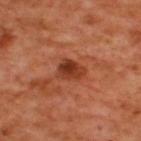Assessment:
The lesion was tiled from a total-body skin photograph and was not biopsied.
Clinical summary:
Longest diameter approximately 3 mm. A 15 mm close-up tile from a total-body photography series done for melanoma screening. Located on the upper back. The patient is a male approximately 50 years of age. Automated image analysis of the tile measured a lesion area of about 6 mm², an eccentricity of roughly 0.7, and a shape-asymmetry score of about 0.25 (0 = symmetric). The analysis additionally found an average lesion color of about L≈38 a*≈29 b*≈34 (CIELAB), about 12 CIELAB-L* units darker than the surrounding skin, and a lesion-to-skin contrast of about 9 (normalized; higher = more distinct).